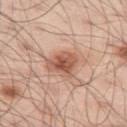Part of a total-body skin-imaging series; this lesion was reviewed on a skin check and was not flagged for biopsy. The lesion is on the left thigh. A 15 mm close-up tile from a total-body photography series done for melanoma screening. The patient is a male aged 53 to 57.